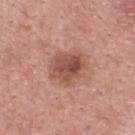A female patient aged 38 to 42. Located on the upper back. Cropped from a whole-body photographic skin survey; the tile spans about 15 mm.